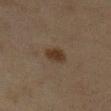notes = catalogued during a skin exam; not biopsied
location = the left lower leg
imaging modality = ~15 mm crop, total-body skin-cancer survey
patient = female, approximately 40 years of age
image-analysis metrics = a footprint of about 4.5 mm², a shape eccentricity near 0.6, and two-axis asymmetry of about 0.15; a classifier nevus-likeness of about 95/100 and a lesion-detection confidence of about 100/100